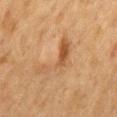  biopsy_status: not biopsied; imaged during a skin examination
  image:
    source: total-body photography crop
    field_of_view_mm: 15
  site: mid back
  patient:
    sex: male
    age_approx: 75
  lesion_size:
    long_diameter_mm_approx: 6.0